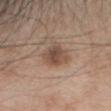{
  "biopsy_status": "not biopsied; imaged during a skin examination",
  "site": "head or neck",
  "patient": {
    "sex": "female",
    "age_approx": 40
  },
  "image": {
    "source": "total-body photography crop",
    "field_of_view_mm": 15
  }
}